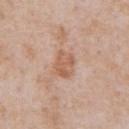No biopsy was performed on this lesion — it was imaged during a full skin examination and was not determined to be concerning. From the chest. This image is a 15 mm lesion crop taken from a total-body photograph. A male patient, in their mid- to late 60s. The tile uses white-light illumination. The lesion-visualizer software estimated a lesion color around L≈59 a*≈21 b*≈31 in CIELAB, about 9 CIELAB-L* units darker than the surrounding skin, and a normalized border contrast of about 6.5. It also reported border irregularity of about 2 on a 0–10 scale, a within-lesion color-variation index near 3/10, and radial color variation of about 1. It also reported a nevus-likeness score of about 0/100 and lesion-presence confidence of about 100/100.